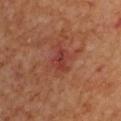Q: Was a biopsy performed?
A: no biopsy performed (imaged during a skin exam)